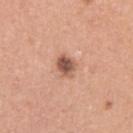Notes:
• follow-up · no biopsy performed (imaged during a skin exam)
• diameter · ~3 mm (longest diameter)
• imaging modality · total-body-photography crop, ~15 mm field of view
• patient · female, aged 33 to 37
• lighting · white-light
• location · the left upper arm